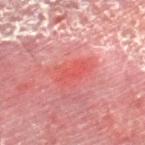biopsy status = imaged on a skin check; not biopsied
acquisition = ~15 mm tile from a whole-body skin photo
body site = the right lower leg
patient = male, in their mid-50s